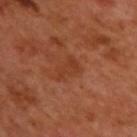A male patient, roughly 50 years of age.
This is a cross-polarized tile.
The lesion is located on the back.
A region of skin cropped from a whole-body photographic capture, roughly 15 mm wide.
The recorded lesion diameter is about 3.5 mm.